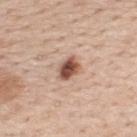{
  "site": "upper back",
  "automated_metrics": {
    "area_mm2_approx": 5.0,
    "eccentricity": 0.7,
    "shape_asymmetry": 0.25,
    "border_irregularity_0_10": 2.0,
    "color_variation_0_10": 5.0,
    "peripheral_color_asymmetry": 1.5
  },
  "image": {
    "source": "total-body photography crop",
    "field_of_view_mm": 15
  },
  "patient": {
    "sex": "male",
    "age_approx": 60
  },
  "lesion_size": {
    "long_diameter_mm_approx": 3.0
  }
}